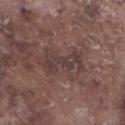{"biopsy_status": "not biopsied; imaged during a skin examination"}